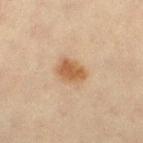<tbp_lesion>
<biopsy_status>not biopsied; imaged during a skin examination</biopsy_status>
<patient>
  <sex>female</sex>
  <age_approx>45</age_approx>
</patient>
<site>left thigh</site>
<lesion_size>
  <long_diameter_mm_approx>3.5</long_diameter_mm_approx>
</lesion_size>
<automated_metrics>
  <area_mm2_approx>7.5</area_mm2_approx>
  <eccentricity>0.7</eccentricity>
  <shape_asymmetry>0.15</shape_asymmetry>
  <border_irregularity_0_10>1.5</border_irregularity_0_10>
  <color_variation_0_10>3.0</color_variation_0_10>
  <peripheral_color_asymmetry>1.0</peripheral_color_asymmetry>
</automated_metrics>
<image>
  <source>total-body photography crop</source>
  <field_of_view_mm>15</field_of_view_mm>
</image>
</tbp_lesion>